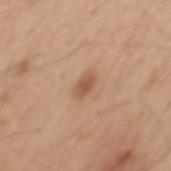Recorded during total-body skin imaging; not selected for excision or biopsy. A male patient, in their mid- to late 40s. Longest diameter approximately 2.5 mm. From the mid back. A lesion tile, about 15 mm wide, cut from a 3D total-body photograph. Imaged with white-light lighting.